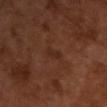Acquisition and patient details: Measured at roughly 2.5 mm in maximum diameter. Automated tile analysis of the lesion measured a footprint of about 2 mm², an outline eccentricity of about 0.95 (0 = round, 1 = elongated), and a symmetry-axis asymmetry near 0.5. The software also gave a border-irregularity index near 5/10 and radial color variation of about 0. The tile uses cross-polarized illumination. This image is a 15 mm lesion crop taken from a total-body photograph. The patient is a male aged 63–67.